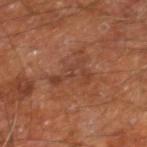The lesion was tiled from a total-body skin photograph and was not biopsied. Automated tile analysis of the lesion measured an area of roughly 6.5 mm² and two-axis asymmetry of about 0.7. And it measured a mean CIELAB color near L≈39 a*≈23 b*≈29, a lesion–skin lightness drop of about 6, and a lesion-to-skin contrast of about 5.5 (normalized; higher = more distinct). It also reported a lesion-detection confidence of about 100/100. The lesion is located on the right leg. About 4.5 mm across. Captured under cross-polarized illumination. A 15 mm crop from a total-body photograph taken for skin-cancer surveillance. The patient is a male aged approximately 60.